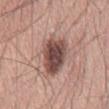Recorded during total-body skin imaging; not selected for excision or biopsy.
From the mid back.
A male subject, aged 58 to 62.
The tile uses white-light illumination.
A lesion tile, about 15 mm wide, cut from a 3D total-body photograph.
Measured at roughly 6.5 mm in maximum diameter.
An algorithmic analysis of the crop reported a footprint of about 17 mm² and a shape-asymmetry score of about 0.25 (0 = symmetric). The analysis additionally found a nevus-likeness score of about 95/100.